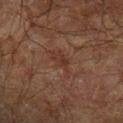No biopsy was performed on this lesion — it was imaged during a full skin examination and was not determined to be concerning.